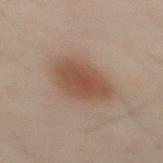Recorded during total-body skin imaging; not selected for excision or biopsy. Imaged with cross-polarized lighting. The lesion-visualizer software estimated an average lesion color of about L≈39 a*≈16 b*≈24 (CIELAB), roughly 8 lightness units darker than nearby skin, and a normalized border contrast of about 7.5. The software also gave a border-irregularity rating of about 1.5/10, internal color variation of about 2.5 on a 0–10 scale, and radial color variation of about 0.5. The lesion's longest dimension is about 4.5 mm. A 15 mm close-up extracted from a 3D total-body photography capture. From the abdomen. A male subject, about 50 years old.